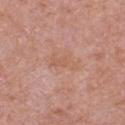The lesion was photographed on a routine skin check and not biopsied; there is no pathology result.
The lesion is located on the right upper arm.
The tile uses white-light illumination.
The patient is a male aged 48 to 52.
Approximately 3.5 mm at its widest.
A 15 mm crop from a total-body photograph taken for skin-cancer surveillance.
An algorithmic analysis of the crop reported a lesion area of about 5 mm², an outline eccentricity of about 0.9 (0 = round, 1 = elongated), and a symmetry-axis asymmetry near 0.45. It also reported a border-irregularity index near 5.5/10 and internal color variation of about 1 on a 0–10 scale. The software also gave an automated nevus-likeness rating near 0 out of 100 and a lesion-detection confidence of about 100/100.